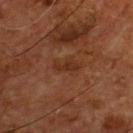Captured during whole-body skin photography for melanoma surveillance; the lesion was not biopsied.
The lesion is on the chest.
A male subject aged around 55.
A 15 mm crop from a total-body photograph taken for skin-cancer surveillance.
The total-body-photography lesion software estimated a lesion area of about 3 mm² and an eccentricity of roughly 0.9.
Measured at roughly 3 mm in maximum diameter.
The tile uses cross-polarized illumination.